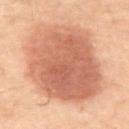<record>
  <biopsy_status>not biopsied; imaged during a skin examination</biopsy_status>
  <patient>
    <sex>male</sex>
    <age_approx>70</age_approx>
  </patient>
  <automated_metrics>
    <area_mm2_approx>65.0</area_mm2_approx>
    <eccentricity>0.4</eccentricity>
    <shape_asymmetry>0.1</shape_asymmetry>
    <cielab_L>46</cielab_L>
    <cielab_a>20</cielab_a>
    <cielab_b>26</cielab_b>
    <vs_skin_darker_L>10.0</vs_skin_darker_L>
    <vs_skin_contrast_norm>8.0</vs_skin_contrast_norm>
    <border_irregularity_0_10>1.5</border_irregularity_0_10>
    <color_variation_0_10>4.0</color_variation_0_10>
    <peripheral_color_asymmetry>1.5</peripheral_color_asymmetry>
    <lesion_detection_confidence_0_100>100</lesion_detection_confidence_0_100>
  </automated_metrics>
  <image>
    <source>total-body photography crop</source>
    <field_of_view_mm>15</field_of_view_mm>
  </image>
  <lesion_size>
    <long_diameter_mm_approx>9.5</long_diameter_mm_approx>
  </lesion_size>
  <site>abdomen</site>
</record>